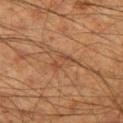This lesion was catalogued during total-body skin photography and was not selected for biopsy. A male subject aged 58 to 62. A 15 mm crop from a total-body photograph taken for skin-cancer surveillance. On the leg. The lesion-visualizer software estimated a lesion area of about 3.5 mm² and a shape-asymmetry score of about 0.4 (0 = symmetric). The software also gave a mean CIELAB color near L≈39 a*≈19 b*≈29, roughly 6 lightness units darker than nearby skin, and a normalized border contrast of about 5. The analysis additionally found a classifier nevus-likeness of about 0/100 and a detector confidence of about 60 out of 100 that the crop contains a lesion. Imaged with cross-polarized lighting. Longest diameter approximately 3 mm.